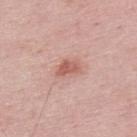The lesion was photographed on a routine skin check and not biopsied; there is no pathology result. The subject is a male aged 48–52. From the upper back. This is a white-light tile. A 15 mm close-up extracted from a 3D total-body photography capture. The lesion-visualizer software estimated internal color variation of about 2 on a 0–10 scale and peripheral color asymmetry of about 1. It also reported an automated nevus-likeness rating near 40 out of 100. Approximately 3 mm at its widest.